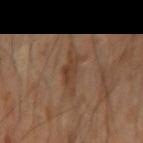notes: imaged on a skin check; not biopsied | automated metrics: a shape eccentricity near 0.9 and a symmetry-axis asymmetry near 0.4; a nevus-likeness score of about 0/100 and lesion-presence confidence of about 100/100 | lighting: cross-polarized illumination | image: total-body-photography crop, ~15 mm field of view | site: the left forearm | lesion diameter: ≈4.5 mm.